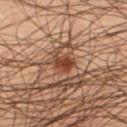Impression:
The lesion was tiled from a total-body skin photograph and was not biopsied.
Image and clinical context:
The patient is a male approximately 45 years of age. The recorded lesion diameter is about 2.5 mm. Cropped from a total-body skin-imaging series; the visible field is about 15 mm. Located on the left thigh. Imaged with cross-polarized lighting.